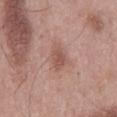Part of a total-body skin-imaging series; this lesion was reviewed on a skin check and was not flagged for biopsy. This is a white-light tile. A close-up tile cropped from a whole-body skin photograph, about 15 mm across. The lesion is on the back. About 3 mm across. The subject is a male aged approximately 55.